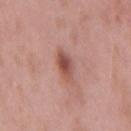Assessment:
No biopsy was performed on this lesion — it was imaged during a full skin examination and was not determined to be concerning.
Acquisition and patient details:
The lesion is on the mid back. The lesion's longest dimension is about 4 mm. Captured under white-light illumination. The patient is a male in their mid-50s. This image is a 15 mm lesion crop taken from a total-body photograph.